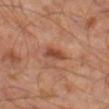Part of a total-body skin-imaging series; this lesion was reviewed on a skin check and was not flagged for biopsy. A 15 mm crop from a total-body photograph taken for skin-cancer surveillance. On the left thigh. The recorded lesion diameter is about 3 mm. A male subject aged approximately 55. The tile uses cross-polarized illumination.